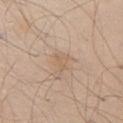Q: Was this lesion biopsied?
A: total-body-photography surveillance lesion; no biopsy
Q: What is the lesion's diameter?
A: ~2.5 mm (longest diameter)
Q: What did automated image analysis measure?
A: a mean CIELAB color near L≈61 a*≈15 b*≈31, roughly 6 lightness units darker than nearby skin, and a lesion-to-skin contrast of about 4.5 (normalized; higher = more distinct); a border-irregularity rating of about 4/10 and a color-variation rating of about 1/10
Q: How was this image acquired?
A: total-body-photography crop, ~15 mm field of view
Q: Patient demographics?
A: male, aged approximately 45
Q: Where on the body is the lesion?
A: the right upper arm